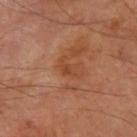biopsy status: no biopsy performed (imaged during a skin exam) | subject: male, aged approximately 70 | acquisition: total-body-photography crop, ~15 mm field of view | site: the leg | automated lesion analysis: a footprint of about 3.5 mm², an outline eccentricity of about 0.8 (0 = round, 1 = elongated), and two-axis asymmetry of about 0.6; a lesion–skin lightness drop of about 6 and a normalized lesion–skin contrast near 5.5; border irregularity of about 6.5 on a 0–10 scale, a within-lesion color-variation index near 2/10, and radial color variation of about 0.5; a nevus-likeness score of about 0/100 and a detector confidence of about 100 out of 100 that the crop contains a lesion | lesion diameter: about 3 mm | lighting: cross-polarized.